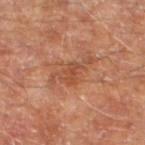The lesion was tiled from a total-body skin photograph and was not biopsied. A male subject, in their 70s. The tile uses cross-polarized illumination. From the leg. Longest diameter approximately 5 mm. Automated image analysis of the tile measured a lesion color around L≈49 a*≈25 b*≈33 in CIELAB, a lesion–skin lightness drop of about 8, and a normalized lesion–skin contrast near 6. And it measured peripheral color asymmetry of about 1. Cropped from a total-body skin-imaging series; the visible field is about 15 mm.